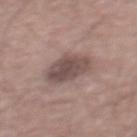Approximately 4.5 mm at its widest. A region of skin cropped from a whole-body photographic capture, roughly 15 mm wide. The lesion-visualizer software estimated a lesion color around L≈49 a*≈16 b*≈19 in CIELAB. The analysis additionally found a border-irregularity index near 2/10, a color-variation rating of about 3.5/10, and peripheral color asymmetry of about 1. The analysis additionally found a classifier nevus-likeness of about 20/100 and a detector confidence of about 100 out of 100 that the crop contains a lesion. Located on the mid back. A male patient in their mid- to late 50s.